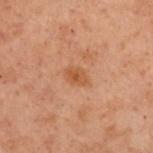The lesion was photographed on a routine skin check and not biopsied; there is no pathology result.
A 15 mm close-up extracted from a 3D total-body photography capture.
Longest diameter approximately 2.5 mm.
Located on the upper back.
Captured under cross-polarized illumination.
A female subject in their mid-50s.
An algorithmic analysis of the crop reported a footprint of about 3.5 mm², an outline eccentricity of about 0.75 (0 = round, 1 = elongated), and a shape-asymmetry score of about 0.2 (0 = symmetric). The analysis additionally found a lesion color around L≈44 a*≈21 b*≈33 in CIELAB, a lesion–skin lightness drop of about 7, and a normalized border contrast of about 6.5. And it measured a border-irregularity index near 1.5/10, a color-variation rating of about 2/10, and a peripheral color-asymmetry measure near 0.5. It also reported a nevus-likeness score of about 25/100.